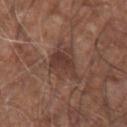{"biopsy_status": "not biopsied; imaged during a skin examination", "site": "right upper arm", "patient": {"sex": "male", "age_approx": 75}, "automated_metrics": {"eccentricity": 0.7, "shape_asymmetry": 0.35}, "image": {"source": "total-body photography crop", "field_of_view_mm": 15}, "lighting": "white-light", "lesion_size": {"long_diameter_mm_approx": 4.5}}